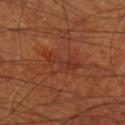Q: Is there a histopathology result?
A: catalogued during a skin exam; not biopsied
Q: What lighting was used for the tile?
A: cross-polarized illumination
Q: What is the anatomic site?
A: the right thigh
Q: What is the imaging modality?
A: ~15 mm tile from a whole-body skin photo
Q: What did automated image analysis measure?
A: lesion-presence confidence of about 100/100
Q: What is the lesion's diameter?
A: ≈4.5 mm
Q: What are the patient's age and sex?
A: male, roughly 70 years of age